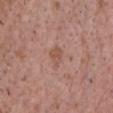<tbp_lesion>
<biopsy_status>not biopsied; imaged during a skin examination</biopsy_status>
<patient>
  <sex>male</sex>
  <age_approx>45</age_approx>
</patient>
<lesion_size>
  <long_diameter_mm_approx>2.5</long_diameter_mm_approx>
</lesion_size>
<site>front of the torso</site>
<image>
  <source>total-body photography crop</source>
  <field_of_view_mm>15</field_of_view_mm>
</image>
<lighting>white-light</lighting>
</tbp_lesion>